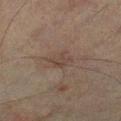Imaged during a routine full-body skin examination; the lesion was not biopsied and no histopathology is available.
A 15 mm crop from a total-body photograph taken for skin-cancer surveillance.
The subject is a male aged 73–77.
Approximately 2.5 mm at its widest.
On the left lower leg.
Automated image analysis of the tile measured a footprint of about 4 mm², an eccentricity of roughly 0.75, and a symmetry-axis asymmetry near 0.4. The analysis additionally found a lesion color around L≈35 a*≈13 b*≈20 in CIELAB and a lesion-to-skin contrast of about 5.5 (normalized; higher = more distinct). The software also gave a classifier nevus-likeness of about 0/100.